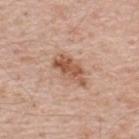Q: Was a biopsy performed?
A: no biopsy performed (imaged during a skin exam)
Q: Patient demographics?
A: male, approximately 50 years of age
Q: Lesion location?
A: the upper back
Q: What is the imaging modality?
A: ~15 mm tile from a whole-body skin photo